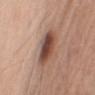workup: total-body-photography surveillance lesion; no biopsy
lesion size: ~4.5 mm (longest diameter)
patient: male, aged 58–62
location: the right upper arm
TBP lesion metrics: a mean CIELAB color near L≈47 a*≈21 b*≈27, a lesion–skin lightness drop of about 14, and a lesion-to-skin contrast of about 10 (normalized; higher = more distinct); a detector confidence of about 100 out of 100 that the crop contains a lesion
image: ~15 mm tile from a whole-body skin photo
lighting: white-light